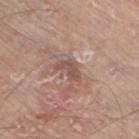workup — catalogued during a skin exam; not biopsied
image-analysis metrics — about 9 CIELAB-L* units darker than the surrounding skin; a nevus-likeness score of about 0/100 and a lesion-detection confidence of about 100/100
image — ~15 mm tile from a whole-body skin photo
illumination — white-light
site — the leg
size — ≈3.5 mm
subject — male, aged 78 to 82